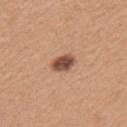Clinical impression: Recorded during total-body skin imaging; not selected for excision or biopsy. Image and clinical context: Located on the left upper arm. Cropped from a whole-body photographic skin survey; the tile spans about 15 mm. The tile uses white-light illumination. The subject is a female roughly 30 years of age. The lesion's longest dimension is about 3 mm.